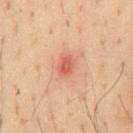| key | value |
|---|---|
| image | ~15 mm crop, total-body skin-cancer survey |
| automated metrics | a lesion area of about 3.5 mm²; a mean CIELAB color near L≈54 a*≈29 b*≈31 and a lesion-to-skin contrast of about 7 (normalized; higher = more distinct); a classifier nevus-likeness of about 0/100 and lesion-presence confidence of about 100/100 |
| subject | male, aged 33–37 |
| lesion size | ~2.5 mm (longest diameter) |
| body site | the chest |